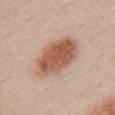Q: Was a biopsy performed?
A: no biopsy performed (imaged during a skin exam)
Q: Who is the patient?
A: female, aged 23–27
Q: What is the imaging modality?
A: ~15 mm crop, total-body skin-cancer survey
Q: Automated lesion metrics?
A: a lesion area of about 22 mm², a shape eccentricity near 0.85, and a symmetry-axis asymmetry near 0.15; an average lesion color of about L≈57 a*≈21 b*≈31 (CIELAB), roughly 14 lightness units darker than nearby skin, and a normalized border contrast of about 9.5
Q: Where on the body is the lesion?
A: the chest
Q: Illumination type?
A: white-light illumination
Q: What is the lesion's diameter?
A: about 7 mm